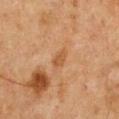follow-up=catalogued during a skin exam; not biopsied
image=~15 mm tile from a whole-body skin photo
lesion diameter=about 2.5 mm
subject=male, aged 48–52
site=the chest